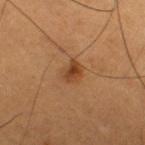No biopsy was performed on this lesion — it was imaged during a full skin examination and was not determined to be concerning.
A 15 mm crop from a total-body photograph taken for skin-cancer surveillance.
On the right thigh.
A male subject aged around 55.
This is a cross-polarized tile.
Measured at roughly 2.5 mm in maximum diameter.